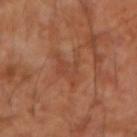follow-up = imaged on a skin check; not biopsied.